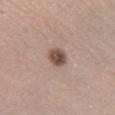<lesion>
<biopsy_status>not biopsied; imaged during a skin examination</biopsy_status>
<image>
  <source>total-body photography crop</source>
  <field_of_view_mm>15</field_of_view_mm>
</image>
<site>right lower leg</site>
<patient>
  <sex>male</sex>
  <age_approx>60</age_approx>
</patient>
<automated_metrics>
  <border_irregularity_0_10>1.5</border_irregularity_0_10>
  <color_variation_0_10>4.0</color_variation_0_10>
  <peripheral_color_asymmetry>1.5</peripheral_color_asymmetry>
  <nevus_likeness_0_100>90</nevus_likeness_0_100>
  <lesion_detection_confidence_0_100>100</lesion_detection_confidence_0_100>
</automated_metrics>
<lighting>white-light</lighting>
</lesion>